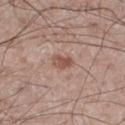image source: total-body-photography crop, ~15 mm field of view | patient: male, roughly 60 years of age | anatomic site: the right thigh | illumination: white-light.